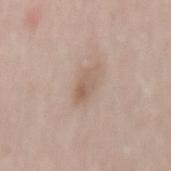Captured during whole-body skin photography for melanoma surveillance; the lesion was not biopsied. A female patient, approximately 30 years of age. The lesion is located on the mid back. A 15 mm close-up extracted from a 3D total-body photography capture. The lesion-visualizer software estimated a normalized lesion–skin contrast near 6. The analysis additionally found a within-lesion color-variation index near 4/10 and radial color variation of about 1.5. Captured under white-light illumination.